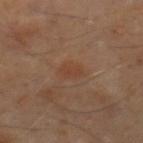• workup · catalogued during a skin exam; not biopsied
• subject · male, aged 58 to 62
• TBP lesion metrics · a footprint of about 4 mm², an eccentricity of roughly 0.8, and a shape-asymmetry score of about 0.15 (0 = symmetric); a mean CIELAB color near L≈38 a*≈19 b*≈27 and a lesion–skin lightness drop of about 4; a classifier nevus-likeness of about 10/100 and a detector confidence of about 100 out of 100 that the crop contains a lesion
• lighting · cross-polarized illumination
• site · the right lower leg
• acquisition · ~15 mm tile from a whole-body skin photo